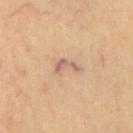Impression:
Imaged during a routine full-body skin examination; the lesion was not biopsied and no histopathology is available.
Image and clinical context:
A region of skin cropped from a whole-body photographic capture, roughly 15 mm wide. From the chest. A male subject, approximately 70 years of age. Imaged with cross-polarized lighting. Automated tile analysis of the lesion measured a mean CIELAB color near L≈59 a*≈18 b*≈27, roughly 9 lightness units darker than nearby skin, and a normalized lesion–skin contrast near 7. And it measured border irregularity of about 6.5 on a 0–10 scale and internal color variation of about 1 on a 0–10 scale. The analysis additionally found a detector confidence of about 90 out of 100 that the crop contains a lesion.